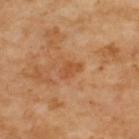Image and clinical context:
A 15 mm close-up extracted from a 3D total-body photography capture. On the upper back. Imaged with cross-polarized lighting.